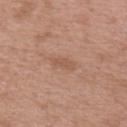Recorded during total-body skin imaging; not selected for excision or biopsy.
Approximately 2.5 mm at its widest.
Imaged with white-light lighting.
The subject is a male aged around 50.
This image is a 15 mm lesion crop taken from a total-body photograph.
The lesion is located on the arm.